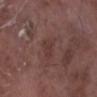{"biopsy_status": "not biopsied; imaged during a skin examination", "patient": {"sex": "male", "age_approx": 75}, "lesion_size": {"long_diameter_mm_approx": 2.5}, "site": "right lower leg", "automated_metrics": {"area_mm2_approx": 3.5, "shape_asymmetry": 0.25, "cielab_L": 36, "cielab_a": 19, "cielab_b": 20, "vs_skin_darker_L": 5.0, "vs_skin_contrast_norm": 4.5, "nevus_likeness_0_100": 0, "lesion_detection_confidence_0_100": 100}, "image": {"source": "total-body photography crop", "field_of_view_mm": 15}, "lighting": "white-light"}